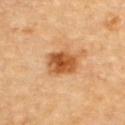Recorded during total-body skin imaging; not selected for excision or biopsy. From the upper back. A male patient, aged 83 or older. A roughly 15 mm field-of-view crop from a total-body skin photograph.